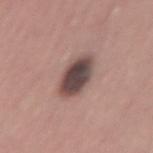Clinical impression:
Recorded during total-body skin imaging; not selected for excision or biopsy.
Image and clinical context:
On the left thigh. This image is a 15 mm lesion crop taken from a total-body photograph. Automated image analysis of the tile measured a mean CIELAB color near L≈45 a*≈16 b*≈18 and a normalized lesion–skin contrast near 12.5. The analysis additionally found a border-irregularity index near 1.5/10 and radial color variation of about 2. The lesion's longest dimension is about 5 mm. The patient is a male aged around 60.